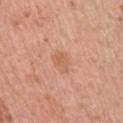Q: Where on the body is the lesion?
A: the right upper arm
Q: What is the lesion's diameter?
A: ~3 mm (longest diameter)
Q: Who is the patient?
A: female, in their 40s
Q: Automated lesion metrics?
A: a mean CIELAB color near L≈61 a*≈24 b*≈34 and a lesion–skin lightness drop of about 6; a classifier nevus-likeness of about 0/100 and lesion-presence confidence of about 100/100
Q: What kind of image is this?
A: total-body-photography crop, ~15 mm field of view